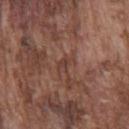workup = total-body-photography surveillance lesion; no biopsy
subject = male, in their mid-70s
lesion diameter = ~2.5 mm (longest diameter)
anatomic site = the chest
acquisition = total-body-photography crop, ~15 mm field of view
tile lighting = white-light